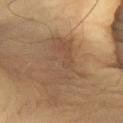Imaged during a routine full-body skin examination; the lesion was not biopsied and no histopathology is available. A 15 mm close-up extracted from a 3D total-body photography capture. A male patient, aged around 65. Longest diameter approximately 6.5 mm. The lesion is located on the chest. An algorithmic analysis of the crop reported a lesion area of about 14 mm², a shape eccentricity near 0.9, and a symmetry-axis asymmetry near 0.5. Captured under cross-polarized illumination.